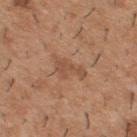follow-up — imaged on a skin check; not biopsied.